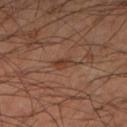notes — no biopsy performed (imaged during a skin exam)
location — the arm
image — 15 mm crop, total-body photography
tile lighting — cross-polarized
patient — male, aged approximately 45